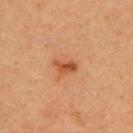Captured during whole-body skin photography for melanoma surveillance; the lesion was not biopsied. The lesion is located on the upper back. This image is a 15 mm lesion crop taken from a total-body photograph. The patient is a female aged 38 to 42.